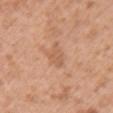Findings:
• biopsy status · catalogued during a skin exam; not biopsied
• patient · female, about 40 years old
• anatomic site · the arm
• imaging modality · ~15 mm crop, total-body skin-cancer survey